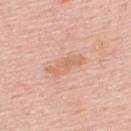{
  "biopsy_status": "not biopsied; imaged during a skin examination"
}